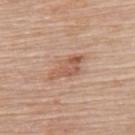Clinical impression: This lesion was catalogued during total-body skin photography and was not selected for biopsy. Image and clinical context: Located on the upper back. The lesion-visualizer software estimated a normalized border contrast of about 6.5. It also reported a border-irregularity rating of about 4/10, a color-variation rating of about 3.5/10, and radial color variation of about 1. And it measured an automated nevus-likeness rating near 50 out of 100 and a lesion-detection confidence of about 100/100. A roughly 15 mm field-of-view crop from a total-body skin photograph. A female subject in their mid-60s. This is a white-light tile. Longest diameter approximately 5 mm.